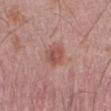Imaged during a routine full-body skin examination; the lesion was not biopsied and no histopathology is available. The lesion is located on the abdomen. The subject is a male about 50 years old. A close-up tile cropped from a whole-body skin photograph, about 15 mm across.